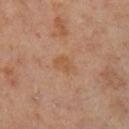Captured during whole-body skin photography for melanoma surveillance; the lesion was not biopsied.
A male patient, aged approximately 60.
A roughly 15 mm field-of-view crop from a total-body skin photograph.
The lesion is on the right lower leg.
The tile uses cross-polarized illumination.